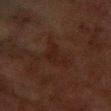Q: Was a biopsy performed?
A: imaged on a skin check; not biopsied
Q: Who is the patient?
A: female, approximately 50 years of age
Q: How large is the lesion?
A: about 3.5 mm
Q: How was this image acquired?
A: ~15 mm tile from a whole-body skin photo
Q: Illumination type?
A: cross-polarized illumination
Q: Where on the body is the lesion?
A: the right forearm